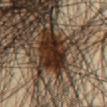Impression: Imaged during a routine full-body skin examination; the lesion was not biopsied and no histopathology is available. Image and clinical context: A close-up tile cropped from a whole-body skin photograph, about 15 mm across. The patient is a male roughly 45 years of age. Captured under cross-polarized illumination. On the abdomen. The recorded lesion diameter is about 4.5 mm.